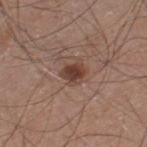Assessment:
Part of a total-body skin-imaging series; this lesion was reviewed on a skin check and was not flagged for biopsy.
Image and clinical context:
The lesion-visualizer software estimated a footprint of about 5 mm², a shape eccentricity near 0.6, and a shape-asymmetry score of about 0.25 (0 = symmetric). The software also gave border irregularity of about 2.5 on a 0–10 scale, internal color variation of about 3.5 on a 0–10 scale, and radial color variation of about 1. The software also gave lesion-presence confidence of about 100/100. The tile uses white-light illumination. A region of skin cropped from a whole-body photographic capture, roughly 15 mm wide. The subject is a male aged 43 to 47. The lesion is on the left thigh. The lesion's longest dimension is about 3 mm.